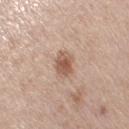Q: Was a biopsy performed?
A: catalogued during a skin exam; not biopsied
Q: What are the patient's age and sex?
A: female, aged 63–67
Q: Lesion size?
A: ~3 mm (longest diameter)
Q: Illumination type?
A: white-light illumination
Q: How was this image acquired?
A: ~15 mm tile from a whole-body skin photo
Q: What is the anatomic site?
A: the arm